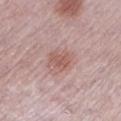<tbp_lesion>
<biopsy_status>not biopsied; imaged during a skin examination</biopsy_status>
<lesion_size>
  <long_diameter_mm_approx>3.0</long_diameter_mm_approx>
</lesion_size>
<lighting>white-light</lighting>
<patient>
  <sex>female</sex>
  <age_approx>65</age_approx>
</patient>
<site>left lower leg</site>
<image>
  <source>total-body photography crop</source>
  <field_of_view_mm>15</field_of_view_mm>
</image>
<automated_metrics>
  <nevus_likeness_0_100>65</nevus_likeness_0_100>
  <lesion_detection_confidence_0_100>100</lesion_detection_confidence_0_100>
</automated_metrics>
</tbp_lesion>